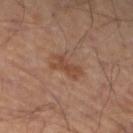<case>
<biopsy_status>not biopsied; imaged during a skin examination</biopsy_status>
<image>
  <source>total-body photography crop</source>
  <field_of_view_mm>15</field_of_view_mm>
</image>
<patient>
  <sex>male</sex>
  <age_approx>50</age_approx>
</patient>
<lighting>cross-polarized</lighting>
<lesion_size>
  <long_diameter_mm_approx>4.0</long_diameter_mm_approx>
</lesion_size>
<site>left leg</site>
<automated_metrics>
  <area_mm2_approx>6.5</area_mm2_approx>
  <eccentricity>0.9</eccentricity>
  <nevus_likeness_0_100>20</nevus_likeness_0_100>
  <lesion_detection_confidence_0_100>100</lesion_detection_confidence_0_100>
</automated_metrics>
</case>